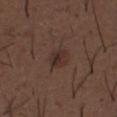<record>
<biopsy_status>not biopsied; imaged during a skin examination</biopsy_status>
<site>abdomen</site>
<image>
  <source>total-body photography crop</source>
  <field_of_view_mm>15</field_of_view_mm>
</image>
<patient>
  <sex>male</sex>
  <age_approx>50</age_approx>
</patient>
</record>